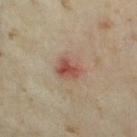Q: Was this lesion biopsied?
A: total-body-photography surveillance lesion; no biopsy
Q: Where on the body is the lesion?
A: the leg
Q: What are the patient's age and sex?
A: female, about 35 years old
Q: What is the imaging modality?
A: total-body-photography crop, ~15 mm field of view
Q: Illumination type?
A: cross-polarized
Q: How large is the lesion?
A: ≈3 mm
Q: Automated lesion metrics?
A: a footprint of about 5 mm² and an outline eccentricity of about 0.65 (0 = round, 1 = elongated)